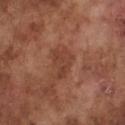{
  "site": "chest",
  "lesion_size": {
    "long_diameter_mm_approx": 3.5
  },
  "patient": {
    "sex": "male",
    "age_approx": 75
  },
  "lighting": "white-light",
  "image": {
    "source": "total-body photography crop",
    "field_of_view_mm": 15
  }
}